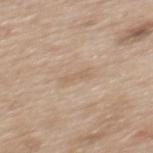workup: no biopsy performed (imaged during a skin exam)
subject: female, in their 40s
anatomic site: the mid back
acquisition: 15 mm crop, total-body photography